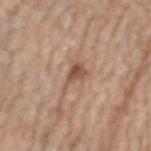Case summary:
– site: the head or neck
– image-analysis metrics: a lesion–skin lightness drop of about 11 and a lesion-to-skin contrast of about 7.5 (normalized; higher = more distinct); a border-irregularity index near 6/10, a within-lesion color-variation index near 3.5/10, and a peripheral color-asymmetry measure near 1.5
– image: ~15 mm tile from a whole-body skin photo
– subject: male, about 70 years old
– tile lighting: white-light illumination